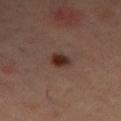The lesion was photographed on a routine skin check and not biopsied; there is no pathology result. Located on the left thigh. A close-up tile cropped from a whole-body skin photograph, about 15 mm across. Captured under cross-polarized illumination. The patient is a female approximately 55 years of age. About 2.5 mm across. The total-body-photography lesion software estimated a footprint of about 3.5 mm², an eccentricity of roughly 0.6, and a symmetry-axis asymmetry near 0.2. The software also gave an average lesion color of about L≈27 a*≈18 b*≈23 (CIELAB) and roughly 11 lightness units darker than nearby skin. The software also gave an automated nevus-likeness rating near 100 out of 100 and a lesion-detection confidence of about 100/100.